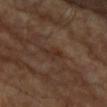Part of a total-body skin-imaging series; this lesion was reviewed on a skin check and was not flagged for biopsy. A 15 mm close-up extracted from a 3D total-body photography capture. The lesion is located on the right forearm. The subject is a male roughly 85 years of age or older. The recorded lesion diameter is about 2.5 mm. The lesion-visualizer software estimated border irregularity of about 4 on a 0–10 scale and peripheral color asymmetry of about 0.5. And it measured a classifier nevus-likeness of about 0/100 and a detector confidence of about 95 out of 100 that the crop contains a lesion.